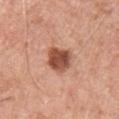* workup — no biopsy performed (imaged during a skin exam)
* illumination — white-light illumination
* location — the chest
* image — total-body-photography crop, ~15 mm field of view
* subject — male, aged 63 to 67
* size — ~3 mm (longest diameter)